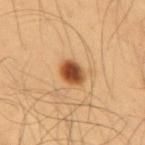site: the mid back
subject: male, in their mid-50s
illumination: cross-polarized
lesion size: ~3 mm (longest diameter)
acquisition: ~15 mm tile from a whole-body skin photo